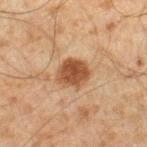No biopsy was performed on this lesion — it was imaged during a full skin examination and was not determined to be concerning.
Cropped from a total-body skin-imaging series; the visible field is about 15 mm.
The lesion is on the right lower leg.
The subject is a male aged 43 to 47.
Measured at roughly 3.5 mm in maximum diameter.